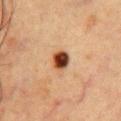biopsy status = no biopsy performed (imaged during a skin exam); location = the chest; imaging modality = 15 mm crop, total-body photography; subject = male, aged approximately 50.